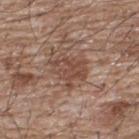The lesion was tiled from a total-body skin photograph and was not biopsied.
An algorithmic analysis of the crop reported an area of roughly 10 mm², an outline eccentricity of about 0.6 (0 = round, 1 = elongated), and two-axis asymmetry of about 0.35. The software also gave a mean CIELAB color near L≈47 a*≈19 b*≈26, roughly 9 lightness units darker than nearby skin, and a normalized border contrast of about 6.5. The software also gave a border-irregularity index near 4.5/10 and a peripheral color-asymmetry measure near 1. It also reported a lesion-detection confidence of about 100/100.
The tile uses white-light illumination.
A male subject, about 65 years old.
A roughly 15 mm field-of-view crop from a total-body skin photograph.
From the upper back.